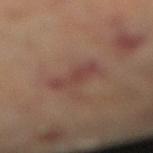Notes:
- workup: total-body-photography surveillance lesion; no biopsy
- illumination: cross-polarized illumination
- patient: female, in their mid- to late 60s
- lesion diameter: about 4.5 mm
- image-analysis metrics: a lesion color around L≈40 a*≈21 b*≈22 in CIELAB and a lesion–skin lightness drop of about 7; a border-irregularity index near 4.5/10 and a color-variation rating of about 1/10
- imaging modality: ~15 mm crop, total-body skin-cancer survey
- location: the left leg